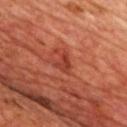Clinical impression: The lesion was tiled from a total-body skin photograph and was not biopsied. Background: The lesion is on the chest. Imaged with cross-polarized lighting. A close-up tile cropped from a whole-body skin photograph, about 15 mm across. A male patient, in their 70s. The recorded lesion diameter is about 2.5 mm.